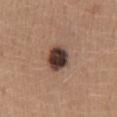Q: Is there a histopathology result?
A: no biopsy performed (imaged during a skin exam)
Q: How was this image acquired?
A: ~15 mm crop, total-body skin-cancer survey
Q: What are the patient's age and sex?
A: female, aged 43 to 47
Q: What did automated image analysis measure?
A: a shape-asymmetry score of about 0.15 (0 = symmetric); a lesion color around L≈37 a*≈16 b*≈21 in CIELAB, about 20 CIELAB-L* units darker than the surrounding skin, and a lesion-to-skin contrast of about 16 (normalized; higher = more distinct)
Q: How was the tile lit?
A: white-light
Q: What is the anatomic site?
A: the lower back
Q: What is the lesion's diameter?
A: ~3.5 mm (longest diameter)